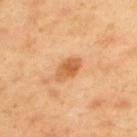biopsy status: total-body-photography surveillance lesion; no biopsy | automated metrics: about 11 CIELAB-L* units darker than the surrounding skin and a normalized border contrast of about 8; a color-variation rating of about 4/10 and radial color variation of about 1.5; a nevus-likeness score of about 85/100 and a lesion-detection confidence of about 100/100 | size: about 3.5 mm | illumination: cross-polarized illumination | anatomic site: the upper back | image: ~15 mm tile from a whole-body skin photo | subject: male, aged 43 to 47.